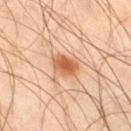Q: Is there a histopathology result?
A: imaged on a skin check; not biopsied
Q: What kind of image is this?
A: ~15 mm tile from a whole-body skin photo
Q: What is the lesion's diameter?
A: about 3 mm
Q: Who is the patient?
A: male, aged 43 to 47
Q: Where on the body is the lesion?
A: the right thigh